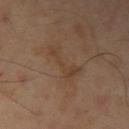Clinical impression:
The lesion was tiled from a total-body skin photograph and was not biopsied.
Background:
The tile uses cross-polarized illumination. An algorithmic analysis of the crop reported border irregularity of about 8.5 on a 0–10 scale and radial color variation of about 0.5. The lesion is located on the left arm. A male subject approximately 50 years of age. A 15 mm close-up tile from a total-body photography series done for melanoma screening. Longest diameter approximately 4.5 mm.